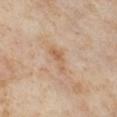• biopsy status: total-body-photography surveillance lesion; no biopsy
• image: total-body-photography crop, ~15 mm field of view
• lesion diameter: ~3 mm (longest diameter)
• subject: female, aged around 55
• body site: the left lower leg